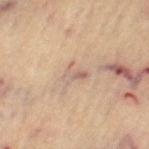This lesion was catalogued during total-body skin photography and was not selected for biopsy.
Approximately 3 mm at its widest.
The subject is a female approximately 65 years of age.
Imaged with cross-polarized lighting.
A region of skin cropped from a whole-body photographic capture, roughly 15 mm wide.
The lesion is on the left thigh.